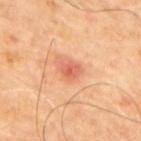Located on the upper back.
The patient is a male approximately 65 years of age.
The lesion-visualizer software estimated internal color variation of about 3 on a 0–10 scale.
This image is a 15 mm lesion crop taken from a total-body photograph.
Measured at roughly 2.5 mm in maximum diameter.